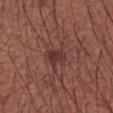Findings:
* patient: male, aged 58–62
* anatomic site: the arm
* lesion diameter: ~4 mm (longest diameter)
* automated metrics: a shape eccentricity near 0.55 and two-axis asymmetry of about 0.45; border irregularity of about 6 on a 0–10 scale and radial color variation of about 1; an automated nevus-likeness rating near 45 out of 100
* illumination: white-light
* acquisition: ~15 mm tile from a whole-body skin photo